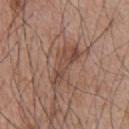Impression: The lesion was photographed on a routine skin check and not biopsied; there is no pathology result. Background: A region of skin cropped from a whole-body photographic capture, roughly 15 mm wide. The tile uses white-light illumination. The subject is a male roughly 65 years of age. Located on the upper back.